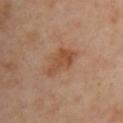follow-up — imaged on a skin check; not biopsied | acquisition — 15 mm crop, total-body photography | illumination — cross-polarized illumination | site — the chest | diameter — about 4.5 mm | subject — female, aged around 40.